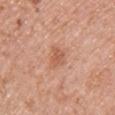Q: Was a biopsy performed?
A: imaged on a skin check; not biopsied
Q: What are the patient's age and sex?
A: female, aged 48–52
Q: Lesion location?
A: the front of the torso
Q: How was the tile lit?
A: white-light
Q: How was this image acquired?
A: total-body-photography crop, ~15 mm field of view
Q: How large is the lesion?
A: ≈3 mm